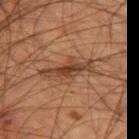follow-up: catalogued during a skin exam; not biopsied
subject: male, aged around 55
anatomic site: the left thigh
image: ~15 mm tile from a whole-body skin photo